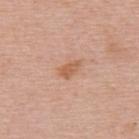subject — female, aged around 50 | illumination — white-light | acquisition — ~15 mm tile from a whole-body skin photo | body site — the upper back | TBP lesion metrics — a lesion color around L≈60 a*≈23 b*≈34 in CIELAB; border irregularity of about 2.5 on a 0–10 scale, a color-variation rating of about 1.5/10, and a peripheral color-asymmetry measure near 0.5 | diameter — ~3 mm (longest diameter).